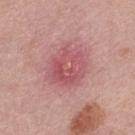<record>
  <lighting>white-light</lighting>
  <lesion_size>
    <long_diameter_mm_approx>4.0</long_diameter_mm_approx>
  </lesion_size>
  <site>mid back</site>
  <automated_metrics>
    <border_irregularity_0_10>2.0</border_irregularity_0_10>
    <color_variation_0_10>5.5</color_variation_0_10>
    <peripheral_color_asymmetry>2.0</peripheral_color_asymmetry>
    <nevus_likeness_0_100>0</nevus_likeness_0_100>
    <lesion_detection_confidence_0_100>100</lesion_detection_confidence_0_100>
  </automated_metrics>
  <image>
    <source>total-body photography crop</source>
    <field_of_view_mm>15</field_of_view_mm>
  </image>
  <patient>
    <sex>male</sex>
    <age_approx>45</age_approx>
  </patient>
</record>